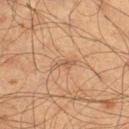Q: Is there a histopathology result?
A: catalogued during a skin exam; not biopsied
Q: What is the anatomic site?
A: the leg
Q: What kind of image is this?
A: ~15 mm tile from a whole-body skin photo
Q: What are the patient's age and sex?
A: male, aged approximately 60